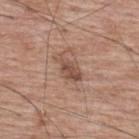Captured during whole-body skin photography for melanoma surveillance; the lesion was not biopsied. Located on the upper back. The lesion's longest dimension is about 3.5 mm. A roughly 15 mm field-of-view crop from a total-body skin photograph. Imaged with white-light lighting. Automated image analysis of the tile measured a mean CIELAB color near L≈50 a*≈19 b*≈27, about 10 CIELAB-L* units darker than the surrounding skin, and a lesion-to-skin contrast of about 7 (normalized; higher = more distinct). The analysis additionally found a border-irregularity index near 3/10, a color-variation rating of about 3/10, and a peripheral color-asymmetry measure near 1. It also reported a nevus-likeness score of about 45/100. A male subject, aged 68–72.